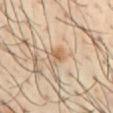Measured at roughly 2.5 mm in maximum diameter. Captured under cross-polarized illumination. The lesion is on the chest. Cropped from a total-body skin-imaging series; the visible field is about 15 mm. A male subject, aged 38–42.